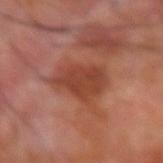Q: Was this lesion biopsied?
A: imaged on a skin check; not biopsied
Q: What are the patient's age and sex?
A: male, aged 68–72
Q: Where on the body is the lesion?
A: the arm
Q: How was this image acquired?
A: 15 mm crop, total-body photography